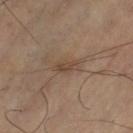Clinical impression:
Imaged during a routine full-body skin examination; the lesion was not biopsied and no histopathology is available.
Acquisition and patient details:
Captured under cross-polarized illumination. On the left thigh. About 3 mm across. A region of skin cropped from a whole-body photographic capture, roughly 15 mm wide. Automated tile analysis of the lesion measured about 7 CIELAB-L* units darker than the surrounding skin and a normalized lesion–skin contrast near 6.